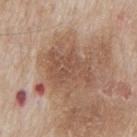Q: Was this lesion biopsied?
A: total-body-photography surveillance lesion; no biopsy
Q: What is the imaging modality?
A: ~15 mm crop, total-body skin-cancer survey
Q: Illumination type?
A: white-light illumination
Q: Lesion location?
A: the mid back
Q: Patient demographics?
A: male, aged 63 to 67
Q: Automated lesion metrics?
A: border irregularity of about 5.5 on a 0–10 scale, internal color variation of about 2.5 on a 0–10 scale, and a peripheral color-asymmetry measure near 1; an automated nevus-likeness rating near 0 out of 100 and lesion-presence confidence of about 95/100
Q: What is the lesion's diameter?
A: about 6 mm